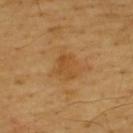Background: The lesion is on the upper back. This image is a 15 mm lesion crop taken from a total-body photograph. The patient is a male aged 63–67.